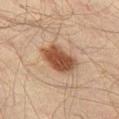Impression: This lesion was catalogued during total-body skin photography and was not selected for biopsy. Clinical summary: On the leg. Cropped from a whole-body photographic skin survey; the tile spans about 15 mm. The tile uses cross-polarized illumination. The patient is a male aged approximately 60. Automated tile analysis of the lesion measured a lesion color around L≈37 a*≈17 b*≈26 in CIELAB, a lesion–skin lightness drop of about 13, and a normalized border contrast of about 11. The software also gave border irregularity of about 2 on a 0–10 scale and a color-variation rating of about 3/10. It also reported a classifier nevus-likeness of about 95/100 and a lesion-detection confidence of about 100/100.